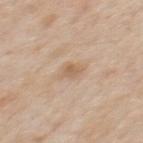Q: Is there a histopathology result?
A: catalogued during a skin exam; not biopsied
Q: Where on the body is the lesion?
A: the back
Q: What is the imaging modality?
A: ~15 mm tile from a whole-body skin photo
Q: Patient demographics?
A: male, aged 58–62
Q: What did automated image analysis measure?
A: a lesion color around L≈61 a*≈16 b*≈32 in CIELAB, about 8 CIELAB-L* units darker than the surrounding skin, and a normalized border contrast of about 6
Q: What lighting was used for the tile?
A: white-light illumination